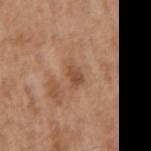Clinical impression:
Captured during whole-body skin photography for melanoma surveillance; the lesion was not biopsied.